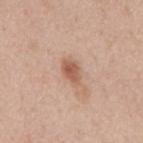notes = catalogued during a skin exam; not biopsied | site = the mid back | subject = male, about 50 years old | imaging modality = ~15 mm crop, total-body skin-cancer survey.